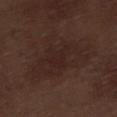Q: What kind of image is this?
A: 15 mm crop, total-body photography
Q: Who is the patient?
A: male, aged approximately 70
Q: Where on the body is the lesion?
A: the right lower leg
Q: What is the lesion's diameter?
A: ~8 mm (longest diameter)
Q: What did automated image analysis measure?
A: an area of roughly 26 mm², a shape eccentricity near 0.75, and a symmetry-axis asymmetry near 0.35; an average lesion color of about L≈23 a*≈16 b*≈19 (CIELAB) and a normalized lesion–skin contrast near 5